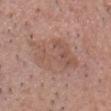Assessment: Captured during whole-body skin photography for melanoma surveillance; the lesion was not biopsied. Clinical summary: The patient is a male approximately 40 years of age. The recorded lesion diameter is about 7 mm. A roughly 15 mm field-of-view crop from a total-body skin photograph. The lesion is on the head or neck. Imaged with white-light lighting.